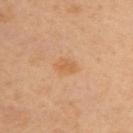subject: female, aged around 40 | illumination: cross-polarized illumination | automated lesion analysis: a lesion color around L≈61 a*≈22 b*≈39 in CIELAB, a lesion–skin lightness drop of about 7, and a lesion-to-skin contrast of about 5.5 (normalized; higher = more distinct); a border-irregularity index near 2/10, a color-variation rating of about 1/10, and a peripheral color-asymmetry measure near 0.5; an automated nevus-likeness rating near 35 out of 100 and a lesion-detection confidence of about 100/100 | location: the upper back | acquisition: total-body-photography crop, ~15 mm field of view | diameter: ~2.5 mm (longest diameter).